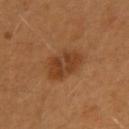Q: Was a biopsy performed?
A: catalogued during a skin exam; not biopsied
Q: Illumination type?
A: cross-polarized illumination
Q: What is the anatomic site?
A: the arm
Q: What kind of image is this?
A: 15 mm crop, total-body photography
Q: Patient demographics?
A: male, in their 40s